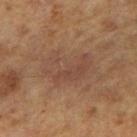Assessment:
Captured during whole-body skin photography for melanoma surveillance; the lesion was not biopsied.
Background:
A male patient, roughly 65 years of age. Longest diameter approximately 6 mm. On the right upper arm. This is a cross-polarized tile. Cropped from a whole-body photographic skin survey; the tile spans about 15 mm.